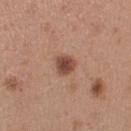The lesion was photographed on a routine skin check and not biopsied; there is no pathology result. The tile uses white-light illumination. The recorded lesion diameter is about 2.5 mm. From the right upper arm. The total-body-photography lesion software estimated a border-irregularity index near 1.5/10, internal color variation of about 3 on a 0–10 scale, and radial color variation of about 0.5. The software also gave a nevus-likeness score of about 90/100 and a detector confidence of about 100 out of 100 that the crop contains a lesion. The subject is a female aged approximately 30. A 15 mm crop from a total-body photograph taken for skin-cancer surveillance.